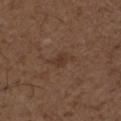Impression: The lesion was photographed on a routine skin check and not biopsied; there is no pathology result. Acquisition and patient details: The patient is a male approximately 50 years of age. This image is a 15 mm lesion crop taken from a total-body photograph. On the left upper arm.